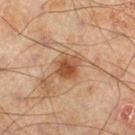workup: imaged on a skin check; not biopsied
automated metrics: an area of roughly 7.5 mm² and a shape-asymmetry score of about 0.2 (0 = symmetric); a lesion color around L≈41 a*≈18 b*≈29 in CIELAB and about 9 CIELAB-L* units darker than the surrounding skin; a border-irregularity rating of about 2/10, a within-lesion color-variation index near 4.5/10, and a peripheral color-asymmetry measure near 1.5
lesion diameter: ≈3 mm
image: total-body-photography crop, ~15 mm field of view
tile lighting: cross-polarized
patient: male, aged 43 to 47
site: the right lower leg